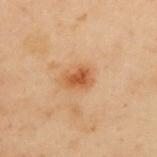Q: Is there a histopathology result?
A: catalogued during a skin exam; not biopsied
Q: What kind of image is this?
A: 15 mm crop, total-body photography
Q: Patient demographics?
A: female, aged approximately 60
Q: Lesion location?
A: the upper back